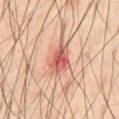Clinical impression: The lesion was tiled from a total-body skin photograph and was not biopsied. Clinical summary: The recorded lesion diameter is about 4.5 mm. The lesion is located on the front of the torso. The patient is a male in their 60s. Automated image analysis of the tile measured an average lesion color of about L≈53 a*≈27 b*≈26 (CIELAB), roughly 12 lightness units darker than nearby skin, and a normalized border contrast of about 8.5. The tile uses cross-polarized illumination. A 15 mm crop from a total-body photograph taken for skin-cancer surveillance.